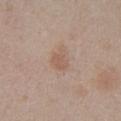Q: Is there a histopathology result?
A: no biopsy performed (imaged during a skin exam)
Q: Patient demographics?
A: female, aged approximately 60
Q: How large is the lesion?
A: ≈3 mm
Q: What is the anatomic site?
A: the leg
Q: Illumination type?
A: white-light
Q: Automated lesion metrics?
A: a footprint of about 5.5 mm², a shape eccentricity near 0.6, and two-axis asymmetry of about 0.3; a border-irregularity index near 3/10, a within-lesion color-variation index near 2/10, and peripheral color asymmetry of about 0.5; a nevus-likeness score of about 10/100 and lesion-presence confidence of about 100/100
Q: How was this image acquired?
A: total-body-photography crop, ~15 mm field of view